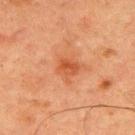Clinical impression:
No biopsy was performed on this lesion — it was imaged during a full skin examination and was not determined to be concerning.
Acquisition and patient details:
Located on the upper back. A male subject, roughly 60 years of age. A 15 mm close-up tile from a total-body photography series done for melanoma screening.